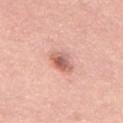Clinical impression: Part of a total-body skin-imaging series; this lesion was reviewed on a skin check and was not flagged for biopsy. Image and clinical context: A roughly 15 mm field-of-view crop from a total-body skin photograph. The lesion is on the mid back. A male patient roughly 60 years of age. Automated image analysis of the tile measured a footprint of about 5 mm², an outline eccentricity of about 0.6 (0 = round, 1 = elongated), and two-axis asymmetry of about 0.25. And it measured an average lesion color of about L≈61 a*≈26 b*≈28 (CIELAB), roughly 13 lightness units darker than nearby skin, and a normalized border contrast of about 8. The recorded lesion diameter is about 2.5 mm. Imaged with white-light lighting.